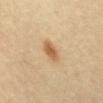<case>
  <biopsy_status>not biopsied; imaged during a skin examination</biopsy_status>
  <site>abdomen</site>
  <lesion_size>
    <long_diameter_mm_approx>3.0</long_diameter_mm_approx>
  </lesion_size>
  <image>
    <source>total-body photography crop</source>
    <field_of_view_mm>15</field_of_view_mm>
  </image>
  <patient>
    <sex>male</sex>
    <age_approx>60</age_approx>
  </patient>
  <automated_metrics>
    <cielab_L>58</cielab_L>
    <cielab_a>19</cielab_a>
    <cielab_b>38</cielab_b>
    <vs_skin_contrast_norm>8.0</vs_skin_contrast_norm>
    <color_variation_0_10>2.5</color_variation_0_10>
    <peripheral_color_asymmetry>0.5</peripheral_color_asymmetry>
  </automated_metrics>
</case>